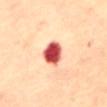{"biopsy_status": "not biopsied; imaged during a skin examination", "lighting": "cross-polarized", "image": {"source": "total-body photography crop", "field_of_view_mm": 15}, "patient": {"sex": "female", "age_approx": 65}, "lesion_size": {"long_diameter_mm_approx": 3.5}, "site": "abdomen"}